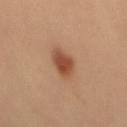  biopsy_status: not biopsied; imaged during a skin examination
  image:
    source: total-body photography crop
    field_of_view_mm: 15
  lighting: cross-polarized
  patient:
    sex: female
    age_approx: 50